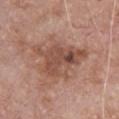{"lesion_size": {"long_diameter_mm_approx": 7.0}, "site": "chest", "image": {"source": "total-body photography crop", "field_of_view_mm": 15}, "patient": {"sex": "male", "age_approx": 80}}